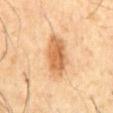Imaged during a routine full-body skin examination; the lesion was not biopsied and no histopathology is available.
On the abdomen.
The lesion's longest dimension is about 5.5 mm.
A 15 mm crop from a total-body photograph taken for skin-cancer surveillance.
A male subject, roughly 60 years of age.